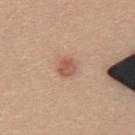| key | value |
|---|---|
| follow-up | no biopsy performed (imaged during a skin exam) |
| location | the upper back |
| subject | female, aged 23–27 |
| image | ~15 mm tile from a whole-body skin photo |